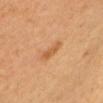Assessment:
Part of a total-body skin-imaging series; this lesion was reviewed on a skin check and was not flagged for biopsy.
Acquisition and patient details:
On the head or neck. Imaged with cross-polarized lighting. A male subject aged approximately 65. Cropped from a whole-body photographic skin survey; the tile spans about 15 mm. The lesion-visualizer software estimated an area of roughly 4.5 mm², an outline eccentricity of about 0.8 (0 = round, 1 = elongated), and two-axis asymmetry of about 0.3. It also reported a lesion–skin lightness drop of about 7 and a normalized lesion–skin contrast near 6. And it measured radial color variation of about 0.5.